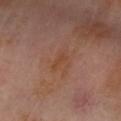A lesion tile, about 15 mm wide, cut from a 3D total-body photograph. The total-body-photography lesion software estimated a classifier nevus-likeness of about 0/100 and a detector confidence of about 100 out of 100 that the crop contains a lesion. A male subject approximately 55 years of age. The tile uses cross-polarized illumination. From the arm.